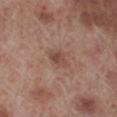Findings:
• follow-up: no biopsy performed (imaged during a skin exam)
• lesion size: ≈3 mm
• image source: ~15 mm tile from a whole-body skin photo
• patient: male, aged approximately 70
• anatomic site: the left lower leg